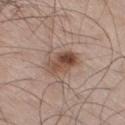patient:
  sex: male
  age_approx: 60
site: right thigh
lighting: white-light
image:
  source: total-body photography crop
  field_of_view_mm: 15
automated_metrics:
  area_mm2_approx: 9.0
  eccentricity: 0.9
  shape_asymmetry: 0.2
  color_variation_0_10: 8.5
  peripheral_color_asymmetry: 4.0
  nevus_likeness_0_100: 90
  lesion_detection_confidence_0_100: 100